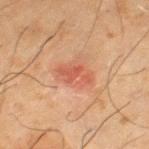Captured during whole-body skin photography for melanoma surveillance; the lesion was not biopsied. A male subject roughly 65 years of age. A region of skin cropped from a whole-body photographic capture, roughly 15 mm wide. The tile uses cross-polarized illumination. Approximately 3.5 mm at its widest. From the left thigh.